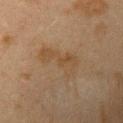Clinical impression:
The lesion was tiled from a total-body skin photograph and was not biopsied.
Image and clinical context:
A female patient, aged around 40. The lesion-visualizer software estimated border irregularity of about 6.5 on a 0–10 scale, a color-variation rating of about 2.5/10, and a peripheral color-asymmetry measure near 1. This is a cross-polarized tile. Cropped from a whole-body photographic skin survey; the tile spans about 15 mm. The lesion is on the left upper arm. Measured at roughly 5.5 mm in maximum diameter.